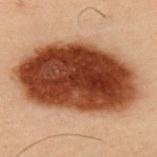Captured during whole-body skin photography for melanoma surveillance; the lesion was not biopsied. About 13 mm across. On the upper back. The lesion-visualizer software estimated a classifier nevus-likeness of about 100/100 and a lesion-detection confidence of about 100/100. A 15 mm close-up tile from a total-body photography series done for melanoma screening. A male subject aged 53–57.